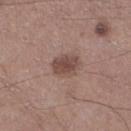biopsy_status: not biopsied; imaged during a skin examination
image:
  source: total-body photography crop
  field_of_view_mm: 15
patient:
  sex: male
  age_approx: 70
site: right lower leg